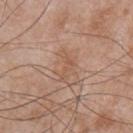Clinical impression: The lesion was tiled from a total-body skin photograph and was not biopsied. Clinical summary: A lesion tile, about 15 mm wide, cut from a 3D total-body photograph. Located on the chest. The subject is a male aged approximately 50.